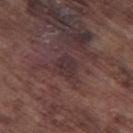  biopsy_status: not biopsied; imaged during a skin examination
  image:
    source: total-body photography crop
    field_of_view_mm: 15
  site: left thigh
  patient:
    sex: male
    age_approx: 75